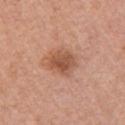Q: Was this lesion biopsied?
A: total-body-photography surveillance lesion; no biopsy
Q: How was this image acquired?
A: ~15 mm crop, total-body skin-cancer survey
Q: Lesion location?
A: the left upper arm
Q: Lesion size?
A: ≈3.5 mm
Q: What are the patient's age and sex?
A: female, aged 58–62
Q: Automated lesion metrics?
A: roughly 11 lightness units darker than nearby skin; a border-irregularity index near 2/10, internal color variation of about 4 on a 0–10 scale, and radial color variation of about 1.5; a nevus-likeness score of about 70/100 and a detector confidence of about 100 out of 100 that the crop contains a lesion
Q: What lighting was used for the tile?
A: white-light illumination